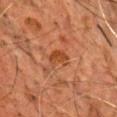Recorded during total-body skin imaging; not selected for excision or biopsy. A close-up tile cropped from a whole-body skin photograph, about 15 mm across. The tile uses cross-polarized illumination. The lesion's longest dimension is about 2.5 mm. On the chest. A male subject aged 63 to 67.